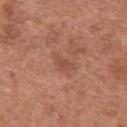Cropped from a total-body skin-imaging series; the visible field is about 15 mm. The tile uses white-light illumination. About 3 mm across. The subject is a male approximately 65 years of age. The lesion is located on the chest.